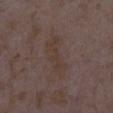The subject is a female aged around 35. This is a white-light tile. The recorded lesion diameter is about 6 mm. On the leg. Cropped from a total-body skin-imaging series; the visible field is about 15 mm.